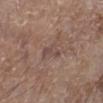Q: Is there a histopathology result?
A: imaged on a skin check; not biopsied
Q: Who is the patient?
A: male, in their mid- to late 60s
Q: What is the lesion's diameter?
A: ≈3 mm
Q: How was the tile lit?
A: white-light illumination
Q: What is the anatomic site?
A: the leg
Q: What kind of image is this?
A: total-body-photography crop, ~15 mm field of view